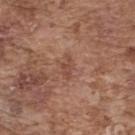Impression: Captured during whole-body skin photography for melanoma surveillance; the lesion was not biopsied.